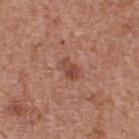Q: Was this lesion biopsied?
A: imaged on a skin check; not biopsied
Q: What is the imaging modality?
A: ~15 mm tile from a whole-body skin photo
Q: Patient demographics?
A: male, approximately 65 years of age
Q: What is the lesion's diameter?
A: about 3 mm
Q: Where on the body is the lesion?
A: the upper back
Q: Illumination type?
A: white-light